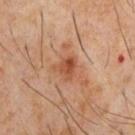On the chest. The lesion-visualizer software estimated a lesion area of about 5 mm², a shape eccentricity near 0.55, and two-axis asymmetry of about 0.45. It also reported a mean CIELAB color near L≈47 a*≈24 b*≈33, about 9 CIELAB-L* units darker than the surrounding skin, and a normalized lesion–skin contrast near 7.5. A roughly 15 mm field-of-view crop from a total-body skin photograph. Captured under cross-polarized illumination. Approximately 3 mm at its widest. The patient is a male aged 58–62.